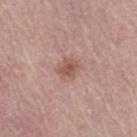Notes:
– biopsy status: total-body-photography surveillance lesion; no biopsy
– image source: 15 mm crop, total-body photography
– image-analysis metrics: an area of roughly 4.5 mm², an outline eccentricity of about 0.5 (0 = round, 1 = elongated), and a shape-asymmetry score of about 0.3 (0 = symmetric); a detector confidence of about 100 out of 100 that the crop contains a lesion
– diameter: ~2.5 mm (longest diameter)
– lighting: white-light
– body site: the right thigh
– patient: female, aged 63 to 67